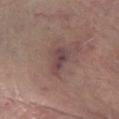The recorded lesion diameter is about 4 mm. The lesion-visualizer software estimated a mean CIELAB color near L≈40 a*≈17 b*≈16 and about 8 CIELAB-L* units darker than the surrounding skin. It also reported lesion-presence confidence of about 95/100. A region of skin cropped from a whole-body photographic capture, roughly 15 mm wide. This is a cross-polarized tile. Located on the left lower leg. A male patient, aged 63–67.